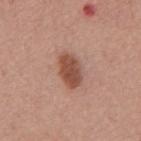– workup: no biopsy performed (imaged during a skin exam)
– lesion diameter: about 4 mm
– imaging modality: total-body-photography crop, ~15 mm field of view
– lighting: white-light
– patient: male, in their mid- to late 50s
– automated metrics: a lesion area of about 8 mm², a shape eccentricity near 0.8, and a shape-asymmetry score of about 0.2 (0 = symmetric); a lesion color around L≈50 a*≈23 b*≈29 in CIELAB, about 13 CIELAB-L* units darker than the surrounding skin, and a normalized lesion–skin contrast near 9.5; a nevus-likeness score of about 95/100 and a lesion-detection confidence of about 100/100
– site: the mid back